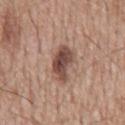Impression: Imaged during a routine full-body skin examination; the lesion was not biopsied and no histopathology is available. Clinical summary: A male patient about 60 years old. A 15 mm close-up tile from a total-body photography series done for melanoma screening. Automated image analysis of the tile measured a border-irregularity rating of about 3.5/10 and internal color variation of about 5 on a 0–10 scale. It also reported a detector confidence of about 100 out of 100 that the crop contains a lesion. On the mid back. Approximately 4.5 mm at its widest.